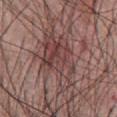Context: The total-body-photography lesion software estimated a lesion area of about 20 mm² and a shape-asymmetry score of about 0.35 (0 = symmetric). The software also gave roughly 9 lightness units darker than nearby skin and a normalized lesion–skin contrast near 7.5. Longest diameter approximately 8.5 mm. From the front of the torso. Cropped from a total-body skin-imaging series; the visible field is about 15 mm. The tile uses white-light illumination. The subject is a male roughly 55 years of age.